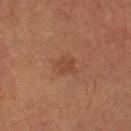{
  "biopsy_status": "not biopsied; imaged during a skin examination",
  "patient": {
    "sex": "male",
    "age_approx": 65
  },
  "image": {
    "source": "total-body photography crop",
    "field_of_view_mm": 15
  },
  "site": "left upper arm"
}